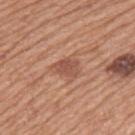notes: no biopsy performed (imaged during a skin exam) | location: the left upper arm | lesion size: about 3.5 mm | image-analysis metrics: a footprint of about 6 mm², a shape eccentricity near 0.75, and a symmetry-axis asymmetry near 0.3; a border-irregularity index near 3/10, a within-lesion color-variation index near 2/10, and radial color variation of about 0.5; an automated nevus-likeness rating near 85 out of 100 and a detector confidence of about 100 out of 100 that the crop contains a lesion | acquisition: ~15 mm crop, total-body skin-cancer survey | subject: male, about 75 years old.